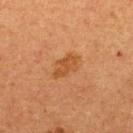Imaged during a routine full-body skin examination; the lesion was not biopsied and no histopathology is available.
The tile uses cross-polarized illumination.
A female patient, approximately 40 years of age.
A roughly 15 mm field-of-view crop from a total-body skin photograph.
On the back.